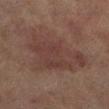– notes: imaged on a skin check; not biopsied
– subject: female, aged 58–62
– site: the left lower leg
– image-analysis metrics: a footprint of about 30 mm², an outline eccentricity of about 0.75 (0 = round, 1 = elongated), and two-axis asymmetry of about 0.4; a mean CIELAB color near L≈33 a*≈16 b*≈19, a lesion–skin lightness drop of about 6, and a normalized lesion–skin contrast near 5.5; a border-irregularity index near 5.5/10, a within-lesion color-variation index near 3/10, and a peripheral color-asymmetry measure near 1; a nevus-likeness score of about 0/100
– illumination: cross-polarized illumination
– lesion diameter: ~8 mm (longest diameter)
– imaging modality: ~15 mm crop, total-body skin-cancer survey